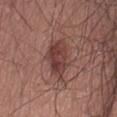Q: Is there a histopathology result?
A: catalogued during a skin exam; not biopsied
Q: What is the lesion's diameter?
A: ≈5 mm
Q: What are the patient's age and sex?
A: male, aged 68 to 72
Q: What is the anatomic site?
A: the lower back
Q: How was this image acquired?
A: total-body-photography crop, ~15 mm field of view
Q: What lighting was used for the tile?
A: white-light illumination
Q: What did automated image analysis measure?
A: a footprint of about 12 mm² and a shape eccentricity near 0.75